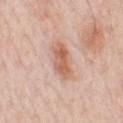The lesion was photographed on a routine skin check and not biopsied; there is no pathology result. Measured at roughly 4.5 mm in maximum diameter. Cropped from a total-body skin-imaging series; the visible field is about 15 mm. The patient is a male about 60 years old. Captured under white-light illumination. The lesion is located on the front of the torso.